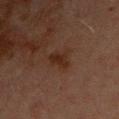Case summary:
- biopsy status: imaged on a skin check; not biopsied
- TBP lesion metrics: an area of roughly 5 mm² and an outline eccentricity of about 0.8 (0 = round, 1 = elongated); border irregularity of about 3.5 on a 0–10 scale and a within-lesion color-variation index near 2/10; an automated nevus-likeness rating near 35 out of 100 and a detector confidence of about 100 out of 100 that the crop contains a lesion
- lesion size: ~3.5 mm (longest diameter)
- image: ~15 mm tile from a whole-body skin photo
- anatomic site: the upper back
- illumination: cross-polarized
- subject: male, about 70 years old